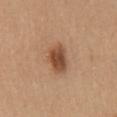Q: Is there a histopathology result?
A: catalogued during a skin exam; not biopsied
Q: What lighting was used for the tile?
A: white-light illumination
Q: Where on the body is the lesion?
A: the mid back
Q: How was this image acquired?
A: ~15 mm tile from a whole-body skin photo
Q: Patient demographics?
A: male, in their 40s
Q: How large is the lesion?
A: ≈4 mm